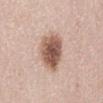{"biopsy_status": "not biopsied; imaged during a skin examination", "lesion_size": {"long_diameter_mm_approx": 4.5}, "site": "abdomen", "lighting": "white-light", "automated_metrics": {"area_mm2_approx": 13.0, "eccentricity": 0.65, "shape_asymmetry": 0.2, "cielab_L": 55, "cielab_a": 20, "cielab_b": 27, "vs_skin_darker_L": 17.0, "nevus_likeness_0_100": 65, "lesion_detection_confidence_0_100": 100}, "patient": {"sex": "female", "age_approx": 50}, "image": {"source": "total-body photography crop", "field_of_view_mm": 15}}